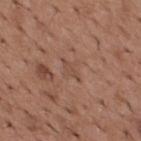{"biopsy_status": "not biopsied; imaged during a skin examination", "site": "back", "lesion_size": {"long_diameter_mm_approx": 2.5}, "image": {"source": "total-body photography crop", "field_of_view_mm": 15}, "automated_metrics": {"border_irregularity_0_10": 5.0, "color_variation_0_10": 0.0, "peripheral_color_asymmetry": 0.0, "nevus_likeness_0_100": 0}, "patient": {"sex": "male", "age_approx": 65}}